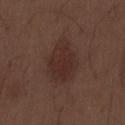This lesion was catalogued during total-body skin photography and was not selected for biopsy. A male subject, aged 68 to 72. About 5.5 mm across. The lesion is on the abdomen. A region of skin cropped from a whole-body photographic capture, roughly 15 mm wide. This is a white-light tile.